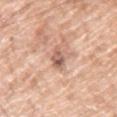biopsy_status: not biopsied; imaged during a skin examination